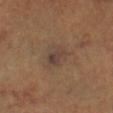Context:
The subject is a female in their mid- to late 60s. Automated tile analysis of the lesion measured an average lesion color of about L≈38 a*≈13 b*≈23 (CIELAB), a lesion–skin lightness drop of about 6, and a lesion-to-skin contrast of about 6.5 (normalized; higher = more distinct). The recorded lesion diameter is about 3.5 mm. A 15 mm crop from a total-body photograph taken for skin-cancer surveillance. Located on the right lower leg.